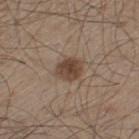Imaged during a routine full-body skin examination; the lesion was not biopsied and no histopathology is available.
This is a white-light tile.
A male patient, approximately 60 years of age.
Longest diameter approximately 3 mm.
This image is a 15 mm lesion crop taken from a total-body photograph.
Located on the left thigh.
Automated image analysis of the tile measured a footprint of about 6 mm² and a shape eccentricity near 0.55. The software also gave a within-lesion color-variation index near 3/10 and a peripheral color-asymmetry measure near 1. The software also gave an automated nevus-likeness rating near 95 out of 100 and a detector confidence of about 100 out of 100 that the crop contains a lesion.